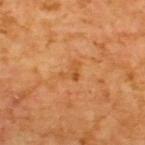No biopsy was performed on this lesion — it was imaged during a full skin examination and was not determined to be concerning.